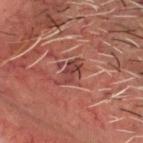workup: no biopsy performed (imaged during a skin exam) | TBP lesion metrics: a color-variation rating of about 3/10 | anatomic site: the head or neck | lighting: cross-polarized | subject: male, aged 63–67 | diameter: ~3.5 mm (longest diameter) | image source: ~15 mm tile from a whole-body skin photo.